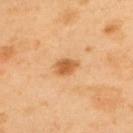Case summary:
– biopsy status: no biopsy performed (imaged during a skin exam)
– location: the upper back
– subject: male, in their 40s
– image source: ~15 mm crop, total-body skin-cancer survey